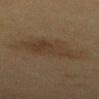Recorded during total-body skin imaging; not selected for excision or biopsy. Captured under cross-polarized illumination. Approximately 8 mm at its widest. A female patient about 50 years old. Automated tile analysis of the lesion measured an outline eccentricity of about 0.8 (0 = round, 1 = elongated) and a symmetry-axis asymmetry near 0.3. It also reported an automated nevus-likeness rating near 20 out of 100 and lesion-presence confidence of about 100/100. A 15 mm crop from a total-body photograph taken for skin-cancer surveillance. The lesion is on the mid back.